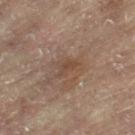{
  "biopsy_status": "not biopsied; imaged during a skin examination",
  "lighting": "cross-polarized",
  "site": "right leg",
  "patient": {
    "sex": "female",
    "age_approx": 80
  },
  "automated_metrics": {
    "vs_skin_darker_L": 6.0,
    "vs_skin_contrast_norm": 5.5,
    "peripheral_color_asymmetry": 0.0,
    "lesion_detection_confidence_0_100": 100
  },
  "image": {
    "source": "total-body photography crop",
    "field_of_view_mm": 15
  }
}